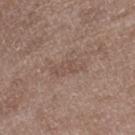workup: imaged on a skin check; not biopsied | lighting: white-light | lesion diameter: about 4 mm | location: the right lower leg | acquisition: 15 mm crop, total-body photography | patient: male, aged approximately 50.